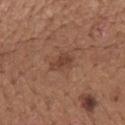Notes:
• workup · catalogued during a skin exam; not biopsied
• patient · male, roughly 65 years of age
• anatomic site · the mid back
• imaging modality · ~15 mm crop, total-body skin-cancer survey
• diameter · ~3 mm (longest diameter)
• automated lesion analysis · about 8 CIELAB-L* units darker than the surrounding skin and a normalized border contrast of about 6.5; a classifier nevus-likeness of about 40/100